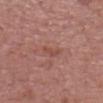The lesion was photographed on a routine skin check and not biopsied; there is no pathology result.
A male subject approximately 70 years of age.
On the head or neck.
A close-up tile cropped from a whole-body skin photograph, about 15 mm across.